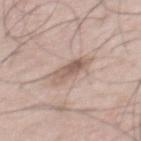Captured under white-light illumination.
An algorithmic analysis of the crop reported an area of roughly 6 mm², a shape eccentricity near 0.9, and a shape-asymmetry score of about 0.2 (0 = symmetric). And it measured an average lesion color of about L≈58 a*≈16 b*≈24 (CIELAB), a lesion–skin lightness drop of about 11, and a normalized border contrast of about 7. It also reported a peripheral color-asymmetry measure near 2. The software also gave lesion-presence confidence of about 100/100.
Measured at roughly 4 mm in maximum diameter.
Located on the mid back.
A lesion tile, about 15 mm wide, cut from a 3D total-body photograph.
The subject is a male approximately 55 years of age.